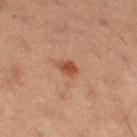Findings:
* workup: total-body-photography surveillance lesion; no biopsy
* anatomic site: the left thigh
* patient: female
* tile lighting: cross-polarized illumination
* automated metrics: a footprint of about 3 mm², an outline eccentricity of about 0.8 (0 = round, 1 = elongated), and a symmetry-axis asymmetry near 0.2; a lesion color around L≈50 a*≈26 b*≈35 in CIELAB and roughly 11 lightness units darker than nearby skin; border irregularity of about 2 on a 0–10 scale, a within-lesion color-variation index near 2.5/10, and a peripheral color-asymmetry measure near 1; an automated nevus-likeness rating near 90 out of 100
* image: ~15 mm crop, total-body skin-cancer survey